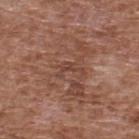The lesion is on the back.
Automated tile analysis of the lesion measured an area of roughly 2.5 mm² and an outline eccentricity of about 0.85 (0 = round, 1 = elongated). The analysis additionally found a classifier nevus-likeness of about 0/100 and a lesion-detection confidence of about 65/100.
This is a white-light tile.
Cropped from a total-body skin-imaging series; the visible field is about 15 mm.
A male patient, in their mid- to late 70s.
Measured at roughly 3 mm in maximum diameter.